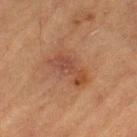Q: Was this lesion biopsied?
A: no biopsy performed (imaged during a skin exam)
Q: Patient demographics?
A: male, in their mid- to late 80s or older
Q: Lesion size?
A: ≈4.5 mm
Q: What is the anatomic site?
A: the left thigh
Q: What is the imaging modality?
A: total-body-photography crop, ~15 mm field of view
Q: How was the tile lit?
A: cross-polarized illumination